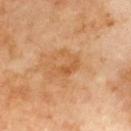The recorded lesion diameter is about 4 mm. Imaged with cross-polarized lighting. The total-body-photography lesion software estimated an outline eccentricity of about 0.65 (0 = round, 1 = elongated) and a shape-asymmetry score of about 0.6 (0 = symmetric). The software also gave a mean CIELAB color near L≈56 a*≈23 b*≈41, about 7 CIELAB-L* units darker than the surrounding skin, and a normalized border contrast of about 5.5. The analysis additionally found border irregularity of about 7.5 on a 0–10 scale, a color-variation rating of about 3/10, and peripheral color asymmetry of about 1. It also reported an automated nevus-likeness rating near 0 out of 100 and lesion-presence confidence of about 100/100. From the upper back. A 15 mm close-up tile from a total-body photography series done for melanoma screening. A male patient, about 70 years old.